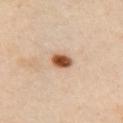Clinical impression: Imaged during a routine full-body skin examination; the lesion was not biopsied and no histopathology is available. Acquisition and patient details: Longest diameter approximately 2.5 mm. A female patient, approximately 40 years of age. A region of skin cropped from a whole-body photographic capture, roughly 15 mm wide. The lesion is located on the left arm.